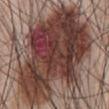Impression:
The lesion was tiled from a total-body skin photograph and was not biopsied.